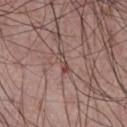Notes:
– biopsy status: total-body-photography surveillance lesion; no biopsy
– lesion diameter: about 3 mm
– body site: the chest
– acquisition: 15 mm crop, total-body photography
– illumination: white-light illumination
– image-analysis metrics: a footprint of about 2.5 mm² and two-axis asymmetry of about 0.4; an average lesion color of about L≈46 a*≈19 b*≈21 (CIELAB), about 8 CIELAB-L* units darker than the surrounding skin, and a normalized border contrast of about 6.5; a classifier nevus-likeness of about 0/100 and a lesion-detection confidence of about 70/100
– subject: male, about 55 years old